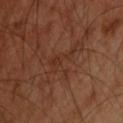{"biopsy_status": "not biopsied; imaged during a skin examination", "patient": {"sex": "male", "age_approx": 45}, "site": "upper back", "image": {"source": "total-body photography crop", "field_of_view_mm": 15}}